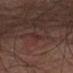Recorded during total-body skin imaging; not selected for excision or biopsy. A male subject aged 73–77. The lesion is on the left upper arm. An algorithmic analysis of the crop reported a lesion color around L≈23 a*≈17 b*≈16 in CIELAB. It also reported border irregularity of about 2.5 on a 0–10 scale, a within-lesion color-variation index near 1/10, and a peripheral color-asymmetry measure near 0. It also reported an automated nevus-likeness rating near 0 out of 100. This is a cross-polarized tile. The lesion's longest dimension is about 3 mm. A roughly 15 mm field-of-view crop from a total-body skin photograph.